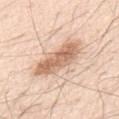Case summary:
• biopsy status · total-body-photography surveillance lesion; no biopsy
• lesion size · ≈7 mm
• image source · total-body-photography crop, ~15 mm field of view
• patient · male, aged around 80
• tile lighting · white-light illumination
• site · the front of the torso